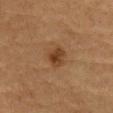No biopsy was performed on this lesion — it was imaged during a full skin examination and was not determined to be concerning.
A male subject aged approximately 85 or older.
This is a cross-polarized tile.
Measured at roughly 2.5 mm in maximum diameter.
Cropped from a total-body skin-imaging series; the visible field is about 15 mm.
The total-body-photography lesion software estimated an average lesion color of about L≈34 a*≈19 b*≈30 (CIELAB), about 9 CIELAB-L* units darker than the surrounding skin, and a lesion-to-skin contrast of about 8.5 (normalized; higher = more distinct). And it measured a border-irregularity rating of about 2.5/10 and a peripheral color-asymmetry measure near 1.
The lesion is on the front of the torso.